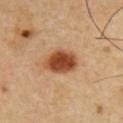• notes — imaged on a skin check; not biopsied
• site — the chest
• TBP lesion metrics — a border-irregularity rating of about 1.5/10, a within-lesion color-variation index near 4.5/10, and radial color variation of about 1.5
• patient — male, aged approximately 55
• acquisition — ~15 mm tile from a whole-body skin photo